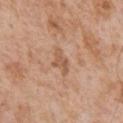Recorded during total-body skin imaging; not selected for excision or biopsy. A 15 mm close-up tile from a total-body photography series done for melanoma screening. About 3 mm across. A male subject, roughly 65 years of age. The lesion is located on the chest. Imaged with white-light lighting. The lesion-visualizer software estimated a mean CIELAB color near L≈56 a*≈21 b*≈33, a lesion–skin lightness drop of about 9, and a normalized lesion–skin contrast near 6. The software also gave a border-irregularity rating of about 4.5/10 and radial color variation of about 0.5.